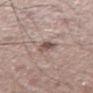workup = total-body-photography surveillance lesion; no biopsy
lesion size = ~2.5 mm (longest diameter)
location = the left thigh
automated lesion analysis = a nevus-likeness score of about 55/100 and a lesion-detection confidence of about 100/100
patient = male, aged 63 to 67
acquisition = ~15 mm tile from a whole-body skin photo
illumination = white-light illumination